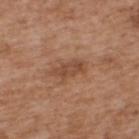Notes:
• biopsy status — no biopsy performed (imaged during a skin exam)
• anatomic site — the upper back
• subject — male, aged 63–67
• image — ~15 mm crop, total-body skin-cancer survey
• lighting — white-light